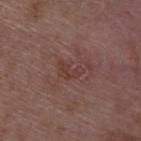Clinical impression:
Imaged during a routine full-body skin examination; the lesion was not biopsied and no histopathology is available.
Clinical summary:
The lesion-visualizer software estimated an area of roughly 3 mm² and an outline eccentricity of about 0.8 (0 = round, 1 = elongated). The tile uses white-light illumination. The subject is a female approximately 45 years of age. This image is a 15 mm lesion crop taken from a total-body photograph. Located on the upper back.